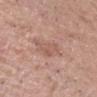Captured during whole-body skin photography for melanoma surveillance; the lesion was not biopsied. The patient is a male aged 38–42. Captured under white-light illumination. Located on the head or neck. The total-body-photography lesion software estimated a lesion area of about 5.5 mm², a shape eccentricity near 0.8, and two-axis asymmetry of about 0.3. The analysis additionally found an average lesion color of about L≈56 a*≈21 b*≈27 (CIELAB), roughly 7 lightness units darker than nearby skin, and a normalized border contrast of about 5. A close-up tile cropped from a whole-body skin photograph, about 15 mm across.